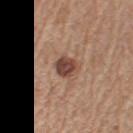This lesion was catalogued during total-body skin photography and was not selected for biopsy.
About 3 mm across.
An algorithmic analysis of the crop reported a footprint of about 6 mm². It also reported a border-irregularity index near 1.5/10 and peripheral color asymmetry of about 1.5.
Cropped from a whole-body photographic skin survey; the tile spans about 15 mm.
The lesion is on the left upper arm.
A male subject, aged around 65.
This is a white-light tile.